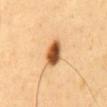Notes:
– workup — no biopsy performed (imaged during a skin exam)
– lighting — cross-polarized
– lesion size — ≈3.5 mm
– image — 15 mm crop, total-body photography
– patient — male, aged around 60
– anatomic site — the abdomen
– image-analysis metrics — an outline eccentricity of about 0.7 (0 = round, 1 = elongated) and a shape-asymmetry score of about 0.15 (0 = symmetric)